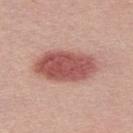Assessment: Captured during whole-body skin photography for melanoma surveillance; the lesion was not biopsied. Background: A male patient roughly 30 years of age. This image is a 15 mm lesion crop taken from a total-body photograph. This is a white-light tile. The lesion-visualizer software estimated an average lesion color of about L≈54 a*≈26 b*≈25 (CIELAB), about 15 CIELAB-L* units darker than the surrounding skin, and a lesion-to-skin contrast of about 9.5 (normalized; higher = more distinct).